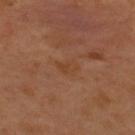The lesion was photographed on a routine skin check and not biopsied; there is no pathology result.
Captured under cross-polarized illumination.
Automated image analysis of the tile measured a border-irregularity rating of about 3.5/10, a color-variation rating of about 1.5/10, and peripheral color asymmetry of about 0.5.
A male subject roughly 50 years of age.
Measured at roughly 2.5 mm in maximum diameter.
The lesion is located on the upper back.
A 15 mm close-up tile from a total-body photography series done for melanoma screening.